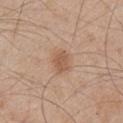Part of a total-body skin-imaging series; this lesion was reviewed on a skin check and was not flagged for biopsy.
The lesion-visualizer software estimated a lesion color around L≈56 a*≈19 b*≈31 in CIELAB, about 9 CIELAB-L* units darker than the surrounding skin, and a lesion-to-skin contrast of about 6.5 (normalized; higher = more distinct).
A 15 mm close-up extracted from a 3D total-body photography capture.
The lesion is on the right upper arm.
The recorded lesion diameter is about 3 mm.
The patient is a male roughly 45 years of age.
Captured under white-light illumination.